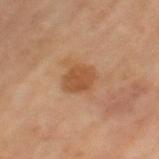– notes · no biopsy performed (imaged during a skin exam)
– anatomic site · the left thigh
– patient · female, about 70 years old
– imaging modality · 15 mm crop, total-body photography